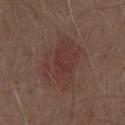Clinical impression: The lesion was tiled from a total-body skin photograph and was not biopsied. Image and clinical context: An algorithmic analysis of the crop reported an area of roughly 20 mm² and an outline eccentricity of about 0.75 (0 = round, 1 = elongated). And it measured about 6 CIELAB-L* units darker than the surrounding skin and a lesion-to-skin contrast of about 5.5 (normalized; higher = more distinct). It also reported a lesion-detection confidence of about 100/100. A 15 mm close-up tile from a total-body photography series done for melanoma screening. A male subject approximately 70 years of age. On the chest. Imaged with white-light lighting. Approximately 6.5 mm at its widest.